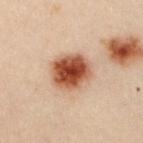Q: Is there a histopathology result?
A: imaged on a skin check; not biopsied
Q: What did automated image analysis measure?
A: an outline eccentricity of about 0.5 (0 = round, 1 = elongated); border irregularity of about 1 on a 0–10 scale, internal color variation of about 6 on a 0–10 scale, and a peripheral color-asymmetry measure near 1.5; an automated nevus-likeness rating near 100 out of 100
Q: Where on the body is the lesion?
A: the chest
Q: What are the patient's age and sex?
A: female, approximately 30 years of age
Q: What is the lesion's diameter?
A: ≈4.5 mm
Q: What lighting was used for the tile?
A: cross-polarized illumination
Q: What is the imaging modality?
A: total-body-photography crop, ~15 mm field of view